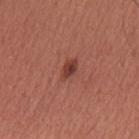No biopsy was performed on this lesion — it was imaged during a full skin examination and was not determined to be concerning.
Captured under white-light illumination.
Approximately 2.5 mm at its widest.
A male subject, aged 43 to 47.
From the chest.
A close-up tile cropped from a whole-body skin photograph, about 15 mm across.